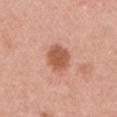Q: Was this lesion biopsied?
A: total-body-photography surveillance lesion; no biopsy
Q: Who is the patient?
A: female, roughly 45 years of age
Q: What did automated image analysis measure?
A: an area of roughly 8.5 mm², a shape eccentricity near 0.55, and two-axis asymmetry of about 0.15; a lesion color around L≈56 a*≈25 b*≈33 in CIELAB, about 13 CIELAB-L* units darker than the surrounding skin, and a lesion-to-skin contrast of about 8.5 (normalized; higher = more distinct); border irregularity of about 1.5 on a 0–10 scale and a peripheral color-asymmetry measure near 1; lesion-presence confidence of about 100/100
Q: How large is the lesion?
A: about 3.5 mm
Q: Lesion location?
A: the left upper arm
Q: What is the imaging modality?
A: ~15 mm crop, total-body skin-cancer survey
Q: Illumination type?
A: white-light